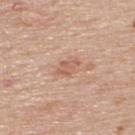follow-up: no biopsy performed (imaged during a skin exam); patient: male, in their mid-70s; site: the upper back; lighting: white-light illumination; image: total-body-photography crop, ~15 mm field of view; lesion size: ~2.5 mm (longest diameter).